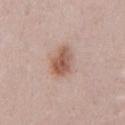Clinical impression:
No biopsy was performed on this lesion — it was imaged during a full skin examination and was not determined to be concerning.
Context:
A 15 mm crop from a total-body photograph taken for skin-cancer surveillance. A male subject, aged approximately 40. Imaged with white-light lighting. Measured at roughly 4 mm in maximum diameter. The lesion is on the chest. Automated image analysis of the tile measured an area of roughly 8.5 mm², an outline eccentricity of about 0.75 (0 = round, 1 = elongated), and a shape-asymmetry score of about 0.2 (0 = symmetric). The analysis additionally found a mean CIELAB color near L≈57 a*≈20 b*≈27 and about 12 CIELAB-L* units darker than the surrounding skin. The analysis additionally found a border-irregularity rating of about 2/10, internal color variation of about 4.5 on a 0–10 scale, and radial color variation of about 1.5.